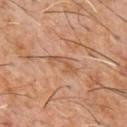notes = catalogued during a skin exam; not biopsied
size = about 4 mm
patient = male, aged around 60
image-analysis metrics = a border-irregularity rating of about 7.5/10, a within-lesion color-variation index near 0/10, and radial color variation of about 0
illumination = cross-polarized
body site = the chest
image = ~15 mm crop, total-body skin-cancer survey